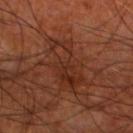| feature | finding |
|---|---|
| notes | total-body-photography surveillance lesion; no biopsy |
| subject | male, roughly 70 years of age |
| site | the left lower leg |
| imaging modality | 15 mm crop, total-body photography |